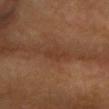{
  "biopsy_status": "not biopsied; imaged during a skin examination",
  "site": "head or neck",
  "automated_metrics": {
    "cielab_L": 37,
    "cielab_a": 21,
    "cielab_b": 30,
    "vs_skin_contrast_norm": 5.0,
    "nevus_likeness_0_100": 10,
    "lesion_detection_confidence_0_100": 100
  },
  "patient": {
    "sex": "female",
    "age_approx": 75
  },
  "image": {
    "source": "total-body photography crop",
    "field_of_view_mm": 15
  },
  "lighting": "cross-polarized",
  "lesion_size": {
    "long_diameter_mm_approx": 3.0
  }
}